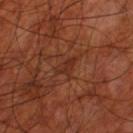Impression: The lesion was photographed on a routine skin check and not biopsied; there is no pathology result. Image and clinical context: A region of skin cropped from a whole-body photographic capture, roughly 15 mm wide. A male patient approximately 70 years of age. Measured at roughly 3 mm in maximum diameter. On the leg. Imaged with cross-polarized lighting.